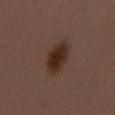Q: Is there a histopathology result?
A: catalogued during a skin exam; not biopsied
Q: Lesion size?
A: ~5.5 mm (longest diameter)
Q: Who is the patient?
A: female, aged around 50
Q: How was this image acquired?
A: 15 mm crop, total-body photography
Q: Where on the body is the lesion?
A: the mid back